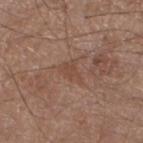Recorded during total-body skin imaging; not selected for excision or biopsy. A male subject aged 58–62. A 15 mm crop from a total-body photograph taken for skin-cancer surveillance. The lesion is located on the left lower leg. Measured at roughly 3 mm in maximum diameter. The tile uses white-light illumination. Automated tile analysis of the lesion measured a lesion area of about 4.5 mm², a shape eccentricity near 0.7, and a shape-asymmetry score of about 0.4 (0 = symmetric). The software also gave a border-irregularity index near 4/10, internal color variation of about 1.5 on a 0–10 scale, and peripheral color asymmetry of about 0.5. It also reported an automated nevus-likeness rating near 0 out of 100 and a lesion-detection confidence of about 95/100.